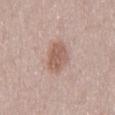Notes:
- workup: catalogued during a skin exam; not biopsied
- anatomic site: the mid back
- diameter: ~4.5 mm (longest diameter)
- acquisition: total-body-photography crop, ~15 mm field of view
- subject: female, approximately 30 years of age
- lighting: white-light illumination